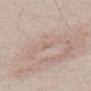{
  "biopsy_status": "not biopsied; imaged during a skin examination",
  "image": {
    "source": "total-body photography crop",
    "field_of_view_mm": 15
  },
  "site": "abdomen",
  "lesion_size": {
    "long_diameter_mm_approx": 1.5
  },
  "automated_metrics": {
    "area_mm2_approx": 1.0,
    "eccentricity": 0.9,
    "shape_asymmetry": 0.3,
    "color_variation_0_10": 0.0,
    "peripheral_color_asymmetry": 0.0,
    "nevus_likeness_0_100": 0,
    "lesion_detection_confidence_0_100": 100
  },
  "patient": {
    "sex": "male",
    "age_approx": 50
  }
}